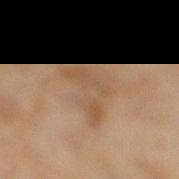<lesion>
<biopsy_status>not biopsied; imaged during a skin examination</biopsy_status>
<site>right lower leg</site>
<patient>
  <sex>female</sex>
  <age_approx>60</age_approx>
</patient>
<image>
  <source>total-body photography crop</source>
  <field_of_view_mm>15</field_of_view_mm>
</image>
</lesion>